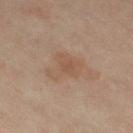Clinical impression:
This lesion was catalogued during total-body skin photography and was not selected for biopsy.
Acquisition and patient details:
This image is a 15 mm lesion crop taken from a total-body photograph. On the right thigh. Measured at roughly 4 mm in maximum diameter. Imaged with cross-polarized lighting. A female subject aged around 50. An algorithmic analysis of the crop reported an eccentricity of roughly 0.7 and a shape-asymmetry score of about 0.5 (0 = symmetric). And it measured a nevus-likeness score of about 0/100 and a lesion-detection confidence of about 100/100.